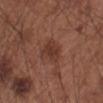The lesion was tiled from a total-body skin photograph and was not biopsied. A male patient aged approximately 55. Approximately 3.5 mm at its widest. From the arm. A lesion tile, about 15 mm wide, cut from a 3D total-body photograph. Imaged with white-light lighting.